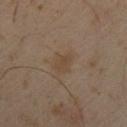A close-up tile cropped from a whole-body skin photograph, about 15 mm across. A male subject aged around 50. The lesion is on the back. Imaged with cross-polarized lighting. Approximately 2.5 mm at its widest.